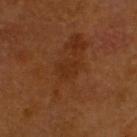This image is a 15 mm lesion crop taken from a total-body photograph. Captured under cross-polarized illumination. Approximately 2.5 mm at its widest. A male patient aged 58–62. Located on the head or neck.